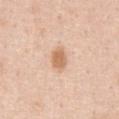  biopsy_status: not biopsied; imaged during a skin examination
  lighting: white-light
  image:
    source: total-body photography crop
    field_of_view_mm: 15
  site: chest
  lesion_size:
    long_diameter_mm_approx: 3.0
  automated_metrics:
    area_mm2_approx: 5.0
    eccentricity: 0.75
    shape_asymmetry: 0.2
    cielab_L: 66
    cielab_a: 21
    cielab_b: 35
    vs_skin_darker_L: 12.0
    vs_skin_contrast_norm: 8.0
    border_irregularity_0_10: 2.0
    peripheral_color_asymmetry: 0.5
  patient:
    sex: male
    age_approx: 50